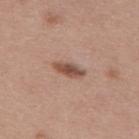Imaged during a routine full-body skin examination; the lesion was not biopsied and no histopathology is available. Measured at roughly 4 mm in maximum diameter. The tile uses white-light illumination. A 15 mm close-up tile from a total-body photography series done for melanoma screening. A female subject, aged 58–62. On the upper back.